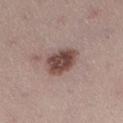<record>
<biopsy_status>not biopsied; imaged during a skin examination</biopsy_status>
<lesion_size>
  <long_diameter_mm_approx>4.0</long_diameter_mm_approx>
</lesion_size>
<lighting>white-light</lighting>
<image>
  <source>total-body photography crop</source>
  <field_of_view_mm>15</field_of_view_mm>
</image>
<site>leg</site>
<automated_metrics>
  <area_mm2_approx>11.0</area_mm2_approx>
  <eccentricity>0.5</eccentricity>
  <shape_asymmetry>0.2</shape_asymmetry>
  <cielab_L>46</cielab_L>
  <cielab_a>18</cielab_a>
  <cielab_b>21</cielab_b>
  <vs_skin_darker_L>14.0</vs_skin_darker_L>
  <border_irregularity_0_10>2.0</border_irregularity_0_10>
  <color_variation_0_10>4.5</color_variation_0_10>
  <peripheral_color_asymmetry>1.5</peripheral_color_asymmetry>
  <lesion_detection_confidence_0_100>100</lesion_detection_confidence_0_100>
</automated_metrics>
<patient>
  <sex>female</sex>
  <age_approx>25</age_approx>
</patient>
</record>